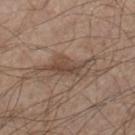workup: no biopsy performed (imaged during a skin exam)
imaging modality: total-body-photography crop, ~15 mm field of view
subject: male, in their mid-40s
location: the right lower leg